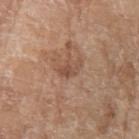<tbp_lesion>
<biopsy_status>not biopsied; imaged during a skin examination</biopsy_status>
<site>right forearm</site>
<automated_metrics>
  <lesion_detection_confidence_0_100>100</lesion_detection_confidence_0_100>
</automated_metrics>
<patient>
  <sex>female</sex>
  <age_approx>75</age_approx>
</patient>
<image>
  <source>total-body photography crop</source>
  <field_of_view_mm>15</field_of_view_mm>
</image>
<lesion_size>
  <long_diameter_mm_approx>3.0</long_diameter_mm_approx>
</lesion_size>
<lighting>white-light</lighting>
</tbp_lesion>